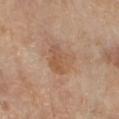Findings:
– follow-up — catalogued during a skin exam; not biopsied
– location — the right forearm
– tile lighting — cross-polarized illumination
– image source — 15 mm crop, total-body photography
– patient — female, aged approximately 55